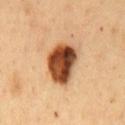Case summary:
* workup · total-body-photography surveillance lesion; no biopsy
* subject · male, roughly 50 years of age
* size · about 5 mm
* imaging modality · ~15 mm crop, total-body skin-cancer survey
* anatomic site · the front of the torso
* lighting · cross-polarized illumination